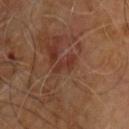Case summary:
* notes — imaged on a skin check; not biopsied
* TBP lesion metrics — a footprint of about 2.5 mm², an eccentricity of roughly 0.95, and two-axis asymmetry of about 0.45; roughly 6 lightness units darker than nearby skin; a border-irregularity index near 5.5/10, a within-lesion color-variation index near 0/10, and peripheral color asymmetry of about 0; a classifier nevus-likeness of about 0/100 and a detector confidence of about 100 out of 100 that the crop contains a lesion
* subject — male, roughly 60 years of age
* diameter — ~3 mm (longest diameter)
* image — ~15 mm crop, total-body skin-cancer survey
* anatomic site — the upper back